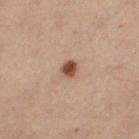The lesion was photographed on a routine skin check and not biopsied; there is no pathology result. The patient is a female aged 43 to 47. A 15 mm crop from a total-body photograph taken for skin-cancer surveillance. From the left thigh. The tile uses cross-polarized illumination.